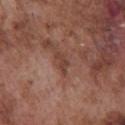The lesion is located on the chest. A region of skin cropped from a whole-body photographic capture, roughly 15 mm wide. Captured under white-light illumination. Automated image analysis of the tile measured border irregularity of about 4 on a 0–10 scale, internal color variation of about 1.5 on a 0–10 scale, and a peripheral color-asymmetry measure near 0.5. Measured at roughly 3 mm in maximum diameter. The patient is a male approximately 75 years of age.